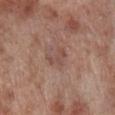workup — no biopsy performed (imaged during a skin exam) | subject — male, in their 70s | lighting — white-light | image — ~15 mm crop, total-body skin-cancer survey | body site — the right lower leg.